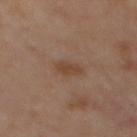biopsy status=no biopsy performed (imaged during a skin exam); illumination=cross-polarized illumination; image source=15 mm crop, total-body photography; anatomic site=the back; lesion diameter=≈2.5 mm.